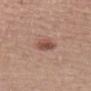Q: Was a biopsy performed?
A: no biopsy performed (imaged during a skin exam)
Q: What kind of image is this?
A: ~15 mm crop, total-body skin-cancer survey
Q: Lesion location?
A: the upper back
Q: Who is the patient?
A: female, about 85 years old or older
Q: What did automated image analysis measure?
A: roughly 11 lightness units darker than nearby skin and a lesion-to-skin contrast of about 8 (normalized; higher = more distinct); a border-irregularity rating of about 3/10, a color-variation rating of about 4/10, and radial color variation of about 1.5
Q: How was the tile lit?
A: white-light
Q: What is the lesion's diameter?
A: ~3.5 mm (longest diameter)